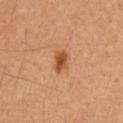Recorded during total-body skin imaging; not selected for excision or biopsy.
The lesion is on the mid back.
A male subject in their 60s.
This image is a 15 mm lesion crop taken from a total-body photograph.